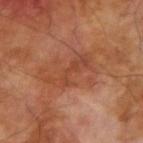Q: Was this lesion biopsied?
A: no biopsy performed (imaged during a skin exam)
Q: Who is the patient?
A: male, roughly 70 years of age
Q: How large is the lesion?
A: ~5.5 mm (longest diameter)
Q: What is the anatomic site?
A: the right upper arm
Q: What kind of image is this?
A: ~15 mm tile from a whole-body skin photo
Q: How was the tile lit?
A: cross-polarized